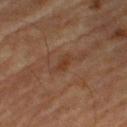Q: Was a biopsy performed?
A: total-body-photography surveillance lesion; no biopsy
Q: What are the patient's age and sex?
A: male, aged 83 or over
Q: Illumination type?
A: cross-polarized
Q: What is the anatomic site?
A: the right thigh
Q: What kind of image is this?
A: ~15 mm tile from a whole-body skin photo